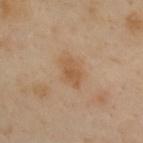image:
  source: total-body photography crop
  field_of_view_mm: 15
site: back
lighting: cross-polarized
patient:
  sex: female
  age_approx: 60
lesion_size:
  long_diameter_mm_approx: 3.5